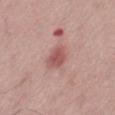Context:
A male subject in their mid- to late 70s. This is a white-light tile. The lesion is located on the right thigh. Automated image analysis of the tile measured a border-irregularity rating of about 2/10 and internal color variation of about 2.5 on a 0–10 scale. The software also gave a nevus-likeness score of about 50/100. A region of skin cropped from a whole-body photographic capture, roughly 15 mm wide. Approximately 3 mm at its widest.